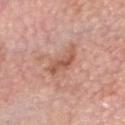Imaged during a routine full-body skin examination; the lesion was not biopsied and no histopathology is available.